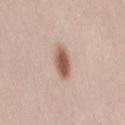Q: Who is the patient?
A: female, approximately 20 years of age
Q: What is the anatomic site?
A: the right thigh
Q: What is the lesion's diameter?
A: ≈4 mm
Q: What is the imaging modality?
A: 15 mm crop, total-body photography
Q: How was the tile lit?
A: white-light illumination